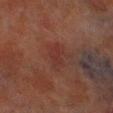Impression: Captured during whole-body skin photography for melanoma surveillance; the lesion was not biopsied. Context: The patient is a male in their 70s. The lesion is on the leg. This image is a 15 mm lesion crop taken from a total-body photograph. Automated image analysis of the tile measured a border-irregularity rating of about 3.5/10, a color-variation rating of about 1.5/10, and a peripheral color-asymmetry measure near 0.5. The software also gave a nevus-likeness score of about 0/100 and a detector confidence of about 100 out of 100 that the crop contains a lesion. This is a cross-polarized tile. Measured at roughly 3 mm in maximum diameter.